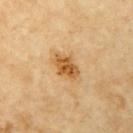Assessment:
Part of a total-body skin-imaging series; this lesion was reviewed on a skin check and was not flagged for biopsy.
Acquisition and patient details:
The recorded lesion diameter is about 3.5 mm. Cropped from a whole-body photographic skin survey; the tile spans about 15 mm. A female patient roughly 70 years of age. The lesion-visualizer software estimated a lesion area of about 6.5 mm², an eccentricity of roughly 0.75, and two-axis asymmetry of about 0.25. The software also gave an average lesion color of about L≈59 a*≈22 b*≈46 (CIELAB) and a normalized border contrast of about 9. The software also gave a nevus-likeness score of about 80/100 and a lesion-detection confidence of about 100/100. From the left upper arm. Captured under cross-polarized illumination.